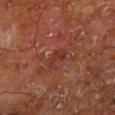biopsy_status: not biopsied; imaged during a skin examination
site: left lower leg
image:
  source: total-body photography crop
  field_of_view_mm: 15
patient:
  sex: male
  age_approx: 65